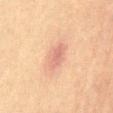The lesion was photographed on a routine skin check and not biopsied; there is no pathology result. This image is a 15 mm lesion crop taken from a total-body photograph. About 3.5 mm across. The lesion is on the front of the torso. The subject is a female about 65 years old. The total-body-photography lesion software estimated an outline eccentricity of about 0.8 (0 = round, 1 = elongated) and two-axis asymmetry of about 0.2.